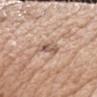Notes:
- follow-up — imaged on a skin check; not biopsied
- patient — male, about 60 years old
- location — the head or neck
- imaging modality — ~15 mm crop, total-body skin-cancer survey
- image-analysis metrics — an area of roughly 4.5 mm², an outline eccentricity of about 0.85 (0 = round, 1 = elongated), and two-axis asymmetry of about 0.3; a classifier nevus-likeness of about 0/100 and a detector confidence of about 95 out of 100 that the crop contains a lesion
- tile lighting — white-light illumination
- lesion diameter — ≈3.5 mm